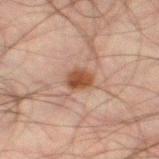  biopsy_status: not biopsied; imaged during a skin examination
  site: left thigh
  patient:
    sex: male
    age_approx: 50
  image:
    source: total-body photography crop
    field_of_view_mm: 15